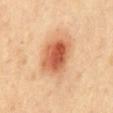Findings:
- histopathologic diagnosis: an intradermal melanocytic nevus — a benign lesion